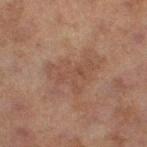Assessment: The lesion was tiled from a total-body skin photograph and was not biopsied. Image and clinical context: Measured at roughly 6 mm in maximum diameter. The tile uses cross-polarized illumination. Located on the left thigh. A female patient, approximately 60 years of age. A region of skin cropped from a whole-body photographic capture, roughly 15 mm wide. The lesion-visualizer software estimated an outline eccentricity of about 0.8 (0 = round, 1 = elongated) and a symmetry-axis asymmetry near 0.5. The analysis additionally found an average lesion color of about L≈42 a*≈18 b*≈25 (CIELAB), roughly 6 lightness units darker than nearby skin, and a normalized lesion–skin contrast near 5.